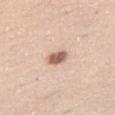  biopsy_status: not biopsied; imaged during a skin examination
  patient:
    sex: female
    age_approx: 30
  lesion_size:
    long_diameter_mm_approx: 3.0
  image:
    source: total-body photography crop
    field_of_view_mm: 15
  site: abdomen
  lighting: white-light